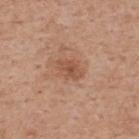Located on the upper back.
The total-body-photography lesion software estimated a footprint of about 4.5 mm², a shape eccentricity near 0.8, and a symmetry-axis asymmetry near 0.3. It also reported a mean CIELAB color near L≈52 a*≈23 b*≈32. It also reported a color-variation rating of about 2.5/10 and radial color variation of about 1. The software also gave a detector confidence of about 100 out of 100 that the crop contains a lesion.
The patient is a male approximately 60 years of age.
A 15 mm crop from a total-body photograph taken for skin-cancer surveillance.
Imaged with white-light lighting.
The recorded lesion diameter is about 3 mm.